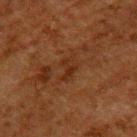{
  "biopsy_status": "not biopsied; imaged during a skin examination",
  "site": "upper back",
  "image": {
    "source": "total-body photography crop",
    "field_of_view_mm": 15
  },
  "lesion_size": {
    "long_diameter_mm_approx": 3.5
  },
  "lighting": "cross-polarized",
  "patient": {
    "sex": "male",
    "age_approx": 60
  },
  "automated_metrics": {
    "cielab_L": 23,
    "cielab_a": 19,
    "cielab_b": 26,
    "vs_skin_darker_L": 5.0,
    "vs_skin_contrast_norm": 5.5
  }
}